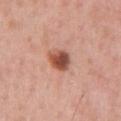* notes · catalogued during a skin exam; not biopsied
* patient · male, roughly 60 years of age
* illumination · white-light
* image source · ~15 mm tile from a whole-body skin photo
* automated lesion analysis · a footprint of about 6 mm², a shape eccentricity near 0.6, and a shape-asymmetry score of about 0.2 (0 = symmetric); an automated nevus-likeness rating near 100 out of 100 and a lesion-detection confidence of about 100/100
* anatomic site · the chest